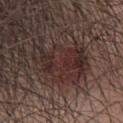Assessment:
The lesion was tiled from a total-body skin photograph and was not biopsied.
Clinical summary:
The lesion is on the head or neck. A lesion tile, about 15 mm wide, cut from a 3D total-body photograph. The patient is a female aged approximately 35.